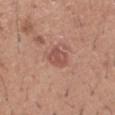The lesion was tiled from a total-body skin photograph and was not biopsied. From the left forearm. The tile uses white-light illumination. Cropped from a total-body skin-imaging series; the visible field is about 15 mm. An algorithmic analysis of the crop reported an average lesion color of about L≈52 a*≈24 b*≈27 (CIELAB), a lesion–skin lightness drop of about 9, and a normalized border contrast of about 6.5. It also reported border irregularity of about 1.5 on a 0–10 scale, a color-variation rating of about 3/10, and peripheral color asymmetry of about 1. And it measured a nevus-likeness score of about 35/100 and a detector confidence of about 100 out of 100 that the crop contains a lesion. The subject is a male aged 38–42. Longest diameter approximately 2.5 mm.